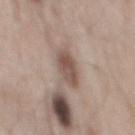- follow-up · total-body-photography surveillance lesion; no biopsy
- location · the mid back
- imaging modality · total-body-photography crop, ~15 mm field of view
- subject · male, aged 53 to 57